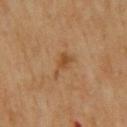notes: total-body-photography surveillance lesion; no biopsy | image-analysis metrics: lesion-presence confidence of about 100/100 | imaging modality: ~15 mm crop, total-body skin-cancer survey | subject: male, roughly 70 years of age | illumination: cross-polarized | size: ≈3.5 mm | body site: the upper back.